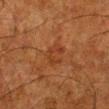The lesion was photographed on a routine skin check and not biopsied; there is no pathology result. The lesion-visualizer software estimated a footprint of about 6 mm², an outline eccentricity of about 0.5 (0 = round, 1 = elongated), and two-axis asymmetry of about 0.3. And it measured a mean CIELAB color near L≈31 a*≈21 b*≈30 and roughly 5 lightness units darker than nearby skin. It also reported border irregularity of about 3.5 on a 0–10 scale, a within-lesion color-variation index near 3.5/10, and a peripheral color-asymmetry measure near 1.5. It also reported a nevus-likeness score of about 0/100 and a lesion-detection confidence of about 100/100. Measured at roughly 3 mm in maximum diameter. A male subject, in their 80s. On the leg. A roughly 15 mm field-of-view crop from a total-body skin photograph. Captured under cross-polarized illumination.